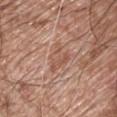Q: Was a biopsy performed?
A: imaged on a skin check; not biopsied
Q: Lesion location?
A: the chest
Q: Who is the patient?
A: male, in their mid- to late 60s
Q: How was this image acquired?
A: 15 mm crop, total-body photography